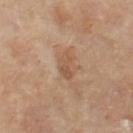| feature | finding |
|---|---|
| notes | no biopsy performed (imaged during a skin exam) |
| acquisition | 15 mm crop, total-body photography |
| lesion diameter | ≈2.5 mm |
| TBP lesion metrics | a mean CIELAB color near L≈51 a*≈18 b*≈31, a lesion–skin lightness drop of about 8, and a lesion-to-skin contrast of about 6 (normalized; higher = more distinct); an automated nevus-likeness rating near 0 out of 100 |
| site | the left thigh |
| subject | female, aged approximately 50 |
| lighting | cross-polarized illumination |